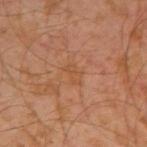| key | value |
|---|---|
| biopsy status | imaged on a skin check; not biopsied |
| location | the left arm |
| illumination | cross-polarized illumination |
| acquisition | ~15 mm crop, total-body skin-cancer survey |
| diameter | about 2.5 mm |
| subject | male, roughly 30 years of age |